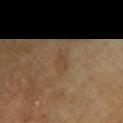{
  "biopsy_status": "not biopsied; imaged during a skin examination",
  "lesion_size": {
    "long_diameter_mm_approx": 2.5
  },
  "site": "right upper arm",
  "patient": {
    "sex": "male",
    "age_approx": 75
  },
  "image": {
    "source": "total-body photography crop",
    "field_of_view_mm": 15
  }
}